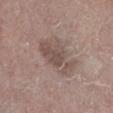Findings:
- biopsy status · total-body-photography surveillance lesion; no biopsy
- size · ~5.5 mm (longest diameter)
- site · the left lower leg
- tile lighting · white-light
- subject · male, in their mid-60s
- imaging modality · ~15 mm crop, total-body skin-cancer survey